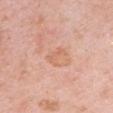Assessment:
The lesion was tiled from a total-body skin photograph and was not biopsied.